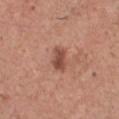Impression: Part of a total-body skin-imaging series; this lesion was reviewed on a skin check and was not flagged for biopsy. Context: The lesion is located on the front of the torso. Imaged with white-light lighting. The recorded lesion diameter is about 3 mm. Automated tile analysis of the lesion measured a footprint of about 4.5 mm² and an eccentricity of roughly 0.8. Cropped from a whole-body photographic skin survey; the tile spans about 15 mm. A male patient, about 45 years old.